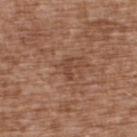Clinical impression: Imaged during a routine full-body skin examination; the lesion was not biopsied and no histopathology is available. Clinical summary: The tile uses white-light illumination. A 15 mm close-up tile from a total-body photography series done for melanoma screening. An algorithmic analysis of the crop reported an area of roughly 2.5 mm² and a shape-asymmetry score of about 0.4 (0 = symmetric). The analysis additionally found an average lesion color of about L≈44 a*≈21 b*≈29 (CIELAB), roughly 7 lightness units darker than nearby skin, and a lesion-to-skin contrast of about 6 (normalized; higher = more distinct). The analysis additionally found a classifier nevus-likeness of about 0/100 and a detector confidence of about 100 out of 100 that the crop contains a lesion. The subject is a male aged 73–77. From the upper back.